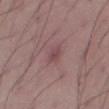The lesion was tiled from a total-body skin photograph and was not biopsied.
Longest diameter approximately 2.5 mm.
A male subject roughly 50 years of age.
From the right thigh.
This image is a 15 mm lesion crop taken from a total-body photograph.